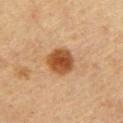Recorded during total-body skin imaging; not selected for excision or biopsy. Measured at roughly 3.5 mm in maximum diameter. An algorithmic analysis of the crop reported a lesion area of about 10 mm², an outline eccentricity of about 0.35 (0 = round, 1 = elongated), and a symmetry-axis asymmetry near 0.1. The analysis additionally found a lesion color around L≈45 a*≈21 b*≈35 in CIELAB, roughly 13 lightness units darker than nearby skin, and a normalized lesion–skin contrast near 10.5. The analysis additionally found a nevus-likeness score of about 100/100. This is a cross-polarized tile. A 15 mm close-up tile from a total-body photography series done for melanoma screening. A male subject, roughly 60 years of age.